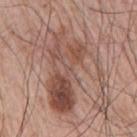Part of a total-body skin-imaging series; this lesion was reviewed on a skin check and was not flagged for biopsy.
A male subject roughly 65 years of age.
A region of skin cropped from a whole-body photographic capture, roughly 15 mm wide.
The lesion is on the right upper arm.
The lesion's longest dimension is about 9.5 mm.